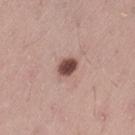Recorded during total-body skin imaging; not selected for excision or biopsy. Approximately 2.5 mm at its widest. A roughly 15 mm field-of-view crop from a total-body skin photograph. The subject is a male approximately 45 years of age. On the left thigh.